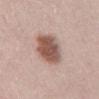Q: Is there a histopathology result?
A: total-body-photography surveillance lesion; no biopsy
Q: How was the tile lit?
A: white-light illumination
Q: How large is the lesion?
A: about 6.5 mm
Q: Automated lesion metrics?
A: a detector confidence of about 100 out of 100 that the crop contains a lesion
Q: Who is the patient?
A: female, in their 30s
Q: What is the imaging modality?
A: ~15 mm tile from a whole-body skin photo
Q: Lesion location?
A: the back